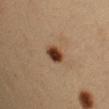Captured during whole-body skin photography for melanoma surveillance; the lesion was not biopsied.
Captured under cross-polarized illumination.
On the arm.
Cropped from a whole-body photographic skin survey; the tile spans about 15 mm.
Approximately 2.5 mm at its widest.
The patient is a female roughly 30 years of age.